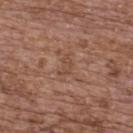follow-up: no biopsy performed (imaged during a skin exam) | subject: female, in their mid-60s | diameter: ~2.5 mm (longest diameter) | image-analysis metrics: roughly 6 lightness units darker than nearby skin and a lesion-to-skin contrast of about 5 (normalized; higher = more distinct) | location: the upper back | tile lighting: white-light illumination | acquisition: 15 mm crop, total-body photography.